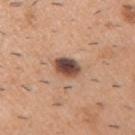<record>
<biopsy_status>not biopsied; imaged during a skin examination</biopsy_status>
<patient>
  <sex>male</sex>
  <age_approx>40</age_approx>
</patient>
<lighting>white-light</lighting>
<image>
  <source>total-body photography crop</source>
  <field_of_view_mm>15</field_of_view_mm>
</image>
<site>right upper arm</site>
<automated_metrics>
  <cielab_L>46</cielab_L>
  <cielab_a>20</cielab_a>
  <cielab_b>25</cielab_b>
  <vs_skin_darker_L>19.0</vs_skin_darker_L>
  <vs_skin_contrast_norm>13.5</vs_skin_contrast_norm>
</automated_metrics>
</record>